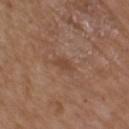{
  "biopsy_status": "not biopsied; imaged during a skin examination",
  "lighting": "white-light",
  "patient": {
    "sex": "male",
    "age_approx": 75
  },
  "site": "arm",
  "image": {
    "source": "total-body photography crop",
    "field_of_view_mm": 15
  }
}